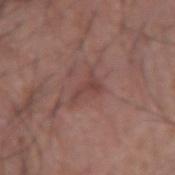<tbp_lesion>
<biopsy_status>not biopsied; imaged during a skin examination</biopsy_status>
<automated_metrics>
  <area_mm2_approx>3.5</area_mm2_approx>
  <eccentricity>0.85</eccentricity>
  <shape_asymmetry>0.65</shape_asymmetry>
  <color_variation_0_10>0.5</color_variation_0_10>
  <peripheral_color_asymmetry>0.0</peripheral_color_asymmetry>
  <nevus_likeness_0_100>0</nevus_likeness_0_100>
  <lesion_detection_confidence_0_100>95</lesion_detection_confidence_0_100>
</automated_metrics>
<image>
  <source>total-body photography crop</source>
  <field_of_view_mm>15</field_of_view_mm>
</image>
<patient>
  <sex>male</sex>
  <age_approx>65</age_approx>
</patient>
<site>right upper arm</site>
<lighting>white-light</lighting>
<lesion_size>
  <long_diameter_mm_approx>3.5</long_diameter_mm_approx>
</lesion_size>
</tbp_lesion>